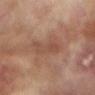biopsy status: no biopsy performed (imaged during a skin exam) | illumination: cross-polarized illumination | body site: the right arm | diameter: ~5 mm (longest diameter) | TBP lesion metrics: a lesion–skin lightness drop of about 6 and a lesion-to-skin contrast of about 5 (normalized; higher = more distinct); a border-irregularity rating of about 4/10, internal color variation of about 3.5 on a 0–10 scale, and peripheral color asymmetry of about 1; a classifier nevus-likeness of about 0/100 and a detector confidence of about 100 out of 100 that the crop contains a lesion | acquisition: ~15 mm crop, total-body skin-cancer survey | subject: female, aged 78 to 82.